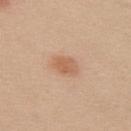| feature | finding |
|---|---|
| follow-up | no biopsy performed (imaged during a skin exam) |
| automated lesion analysis | a lesion area of about 5 mm², an eccentricity of roughly 0.75, and two-axis asymmetry of about 0.2; border irregularity of about 2 on a 0–10 scale, a within-lesion color-variation index near 3/10, and peripheral color asymmetry of about 1; a nevus-likeness score of about 100/100 and lesion-presence confidence of about 100/100 |
| lighting | white-light |
| lesion diameter | ≈3 mm |
| anatomic site | the upper back |
| patient | female, approximately 20 years of age |
| acquisition | 15 mm crop, total-body photography |